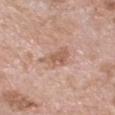Impression: Part of a total-body skin-imaging series; this lesion was reviewed on a skin check and was not flagged for biopsy. Clinical summary: This image is a 15 mm lesion crop taken from a total-body photograph. The total-body-photography lesion software estimated an outline eccentricity of about 0.75 (0 = round, 1 = elongated) and a shape-asymmetry score of about 0.35 (0 = symmetric). And it measured a mean CIELAB color near L≈59 a*≈20 b*≈29, roughly 9 lightness units darker than nearby skin, and a normalized border contrast of about 6. The patient is a female aged approximately 75. Longest diameter approximately 4 mm. The tile uses white-light illumination. From the chest.